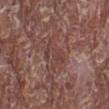Findings:
– follow-up — total-body-photography surveillance lesion; no biopsy
– subject — male, aged around 65
– lighting — white-light
– diameter — ≈4 mm
– image — ~15 mm tile from a whole-body skin photo
– location — the left lower leg